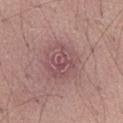Part of a total-body skin-imaging series; this lesion was reviewed on a skin check and was not flagged for biopsy. A roughly 15 mm field-of-view crop from a total-body skin photograph. The total-body-photography lesion software estimated a lesion-detection confidence of about 100/100. Imaged with white-light lighting. The patient is a male aged 63 to 67. Longest diameter approximately 5 mm. From the abdomen.